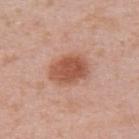notes = total-body-photography surveillance lesion; no biopsy
lesion size = ~5 mm (longest diameter)
image = ~15 mm tile from a whole-body skin photo
anatomic site = the upper back
lighting = white-light illumination
subject = female, approximately 40 years of age